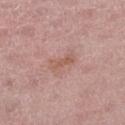No biopsy was performed on this lesion — it was imaged during a full skin examination and was not determined to be concerning.
A female subject, in their mid-40s.
A 15 mm crop from a total-body photograph taken for skin-cancer surveillance.
On the left thigh.
Longest diameter approximately 3 mm.